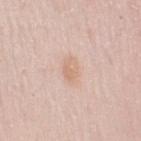• workup — imaged on a skin check; not biopsied
• lesion size — ≈3 mm
• body site — the right upper arm
• imaging modality — total-body-photography crop, ~15 mm field of view
• subject — female, about 30 years old
• tile lighting — white-light illumination
• image-analysis metrics — a lesion color around L≈69 a*≈18 b*≈29 in CIELAB, roughly 7 lightness units darker than nearby skin, and a normalized border contrast of about 5.5; a border-irregularity rating of about 2/10 and a peripheral color-asymmetry measure near 0.5; an automated nevus-likeness rating near 0 out of 100 and lesion-presence confidence of about 100/100